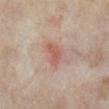<case>
  <biopsy_status>not biopsied; imaged during a skin examination</biopsy_status>
  <image>
    <source>total-body photography crop</source>
    <field_of_view_mm>15</field_of_view_mm>
  </image>
  <site>right lower leg</site>
  <patient>
    <sex>female</sex>
    <age_approx>35</age_approx>
  </patient>
</case>